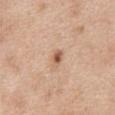Impression: Part of a total-body skin-imaging series; this lesion was reviewed on a skin check and was not flagged for biopsy. Background: The patient is a female approximately 40 years of age. On the abdomen. An algorithmic analysis of the crop reported a border-irregularity rating of about 2/10, a within-lesion color-variation index near 2.5/10, and radial color variation of about 1. A lesion tile, about 15 mm wide, cut from a 3D total-body photograph. The recorded lesion diameter is about 2 mm.